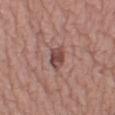Impression:
The lesion was tiled from a total-body skin photograph and was not biopsied.
Image and clinical context:
A female subject approximately 40 years of age. The recorded lesion diameter is about 2.5 mm. Imaged with white-light lighting. The lesion is located on the right thigh. A lesion tile, about 15 mm wide, cut from a 3D total-body photograph. An algorithmic analysis of the crop reported a footprint of about 5 mm² and a shape-asymmetry score of about 0.2 (0 = symmetric). The analysis additionally found a lesion color around L≈46 a*≈21 b*≈22 in CIELAB and a lesion–skin lightness drop of about 11. The software also gave internal color variation of about 3 on a 0–10 scale and a peripheral color-asymmetry measure near 1. The software also gave an automated nevus-likeness rating near 5 out of 100 and lesion-presence confidence of about 100/100.